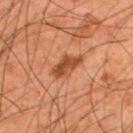biopsy status: total-body-photography surveillance lesion; no biopsy | diameter: ~4 mm (longest diameter) | patient: male, in their mid-40s | anatomic site: the upper back | image: 15 mm crop, total-body photography | lighting: cross-polarized.